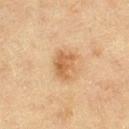Q: Was this lesion biopsied?
A: total-body-photography surveillance lesion; no biopsy
Q: Lesion size?
A: about 3.5 mm
Q: What is the imaging modality?
A: ~15 mm tile from a whole-body skin photo
Q: Where on the body is the lesion?
A: the left thigh
Q: Patient demographics?
A: female, aged 53–57
Q: Automated lesion metrics?
A: a within-lesion color-variation index near 3/10 and radial color variation of about 1